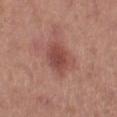biopsy status = catalogued during a skin exam; not biopsied | image-analysis metrics = an area of roughly 10 mm² and an eccentricity of roughly 0.75; an average lesion color of about L≈47 a*≈26 b*≈25 (CIELAB), roughly 10 lightness units darker than nearby skin, and a normalized border contrast of about 7.5; a border-irregularity index near 2/10, a within-lesion color-variation index near 3.5/10, and peripheral color asymmetry of about 1 | patient = female, aged approximately 40 | acquisition = total-body-photography crop, ~15 mm field of view | lesion size = about 4.5 mm | location = the right thigh.